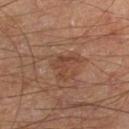The lesion was photographed on a routine skin check and not biopsied; there is no pathology result. A male patient roughly 70 years of age. A region of skin cropped from a whole-body photographic capture, roughly 15 mm wide. Captured under cross-polarized illumination. An algorithmic analysis of the crop reported a footprint of about 5 mm², an eccentricity of roughly 0.6, and two-axis asymmetry of about 0.65. The software also gave a lesion color around L≈41 a*≈21 b*≈29 in CIELAB, a lesion–skin lightness drop of about 6, and a lesion-to-skin contrast of about 5.5 (normalized; higher = more distinct). The software also gave a within-lesion color-variation index near 2/10 and a peripheral color-asymmetry measure near 1. Located on the right lower leg.